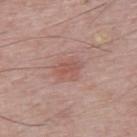Assessment:
Recorded during total-body skin imaging; not selected for excision or biopsy.
Clinical summary:
The lesion-visualizer software estimated a footprint of about 4 mm², an eccentricity of roughly 0.75, and two-axis asymmetry of about 0.25. And it measured border irregularity of about 2.5 on a 0–10 scale and radial color variation of about 0.5. The analysis additionally found a classifier nevus-likeness of about 20/100. The tile uses white-light illumination. A close-up tile cropped from a whole-body skin photograph, about 15 mm across. The subject is a male aged around 65. On the upper back. About 2.5 mm across.